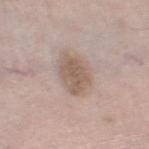- illumination · white-light illumination
- acquisition · total-body-photography crop, ~15 mm field of view
- patient · male, aged 58–62
- location · the leg
- automated metrics · a lesion area of about 9.5 mm² and an outline eccentricity of about 0.8 (0 = round, 1 = elongated); an average lesion color of about L≈57 a*≈15 b*≈24 (CIELAB) and a normalized lesion–skin contrast near 7; a classifier nevus-likeness of about 25/100 and lesion-presence confidence of about 100/100
- lesion size · about 4.5 mm